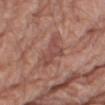<lesion>
  <biopsy_status>not biopsied; imaged during a skin examination</biopsy_status>
  <site>right lower leg</site>
  <lesion_size>
    <long_diameter_mm_approx>4.0</long_diameter_mm_approx>
  </lesion_size>
  <lighting>white-light</lighting>
  <image>
    <source>total-body photography crop</source>
    <field_of_view_mm>15</field_of_view_mm>
  </image>
  <patient>
    <sex>male</sex>
    <age_approx>80</age_approx>
  </patient>
  <automated_metrics>
    <area_mm2_approx>7.0</area_mm2_approx>
    <eccentricity>0.85</eccentricity>
    <cielab_L>48</cielab_L>
    <cielab_a>24</cielab_a>
    <cielab_b>25</cielab_b>
    <vs_skin_darker_L>8.0</vs_skin_darker_L>
    <border_irregularity_0_10>5.5</border_irregularity_0_10>
    <color_variation_0_10>2.5</color_variation_0_10>
  </automated_metrics>
</lesion>